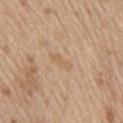follow-up — total-body-photography surveillance lesion; no biopsy | site — the back | imaging modality — total-body-photography crop, ~15 mm field of view | subject — male, in their 70s.